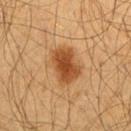Recorded during total-body skin imaging; not selected for excision or biopsy.
The subject is a male aged 58–62.
On the chest.
Captured under cross-polarized illumination.
This image is a 15 mm lesion crop taken from a total-body photograph.